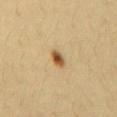This lesion was catalogued during total-body skin photography and was not selected for biopsy. The lesion's longest dimension is about 2.5 mm. Automated image analysis of the tile measured a footprint of about 3.5 mm², an eccentricity of roughly 0.65, and a symmetry-axis asymmetry near 0.15. It also reported a border-irregularity rating of about 1/10, a color-variation rating of about 5.5/10, and a peripheral color-asymmetry measure near 1.5. It also reported a detector confidence of about 100 out of 100 that the crop contains a lesion. The lesion is on the mid back. A lesion tile, about 15 mm wide, cut from a 3D total-body photograph. The tile uses cross-polarized illumination. A male patient roughly 45 years of age.